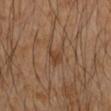Captured during whole-body skin photography for melanoma surveillance; the lesion was not biopsied. An algorithmic analysis of the crop reported a border-irregularity rating of about 4.5/10, a color-variation rating of about 2.5/10, and a peripheral color-asymmetry measure near 1. The software also gave a classifier nevus-likeness of about 5/100 and lesion-presence confidence of about 100/100. The lesion is located on the right forearm. Longest diameter approximately 3 mm. A female subject, aged approximately 45. Cropped from a whole-body photographic skin survey; the tile spans about 15 mm.